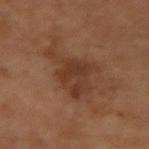Clinical impression: Captured during whole-body skin photography for melanoma surveillance; the lesion was not biopsied. Context: A male subject, approximately 65 years of age. The lesion-visualizer software estimated border irregularity of about 6.5 on a 0–10 scale, a within-lesion color-variation index near 3.5/10, and a peripheral color-asymmetry measure near 1.5. The analysis additionally found a classifier nevus-likeness of about 0/100. About 6 mm across. A lesion tile, about 15 mm wide, cut from a 3D total-body photograph. On the left arm. This is a cross-polarized tile.